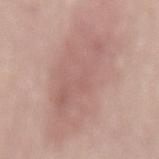Captured during whole-body skin photography for melanoma surveillance; the lesion was not biopsied. Cropped from a total-body skin-imaging series; the visible field is about 15 mm. The lesion is located on the back. A male patient roughly 70 years of age. Measured at roughly 11.5 mm in maximum diameter.